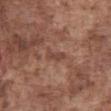The lesion was photographed on a routine skin check and not biopsied; there is no pathology result.